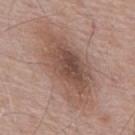Case summary:
* workup — imaged on a skin check; not biopsied
* acquisition — 15 mm crop, total-body photography
* body site — the mid back
* lighting — white-light
* subject — male, roughly 65 years of age
* lesion size — ~11 mm (longest diameter)